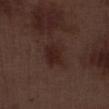Recorded during total-body skin imaging; not selected for excision or biopsy.
A male subject, in their 70s.
The recorded lesion diameter is about 4 mm.
A 15 mm crop from a total-body photograph taken for skin-cancer surveillance.
On the right lower leg.